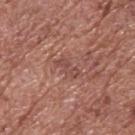| key | value |
|---|---|
| biopsy status | catalogued during a skin exam; not biopsied |
| lesion diameter | about 3 mm |
| anatomic site | the back |
| illumination | white-light illumination |
| image source | total-body-photography crop, ~15 mm field of view |
| subject | male, aged approximately 75 |